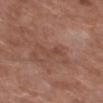<lesion>
<biopsy_status>not biopsied; imaged during a skin examination</biopsy_status>
<lesion_size>
  <long_diameter_mm_approx>3.0</long_diameter_mm_approx>
</lesion_size>
<patient>
  <sex>male</sex>
  <age_approx>65</age_approx>
</patient>
<image>
  <source>total-body photography crop</source>
  <field_of_view_mm>15</field_of_view_mm>
</image>
<automated_metrics>
  <border_irregularity_0_10>6.0</border_irregularity_0_10>
  <color_variation_0_10>0.0</color_variation_0_10>
  <peripheral_color_asymmetry>0.0</peripheral_color_asymmetry>
</automated_metrics>
<site>left thigh</site>
</lesion>